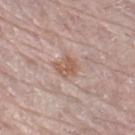<case>
<biopsy_status>not biopsied; imaged during a skin examination</biopsy_status>
<image>
  <source>total-body photography crop</source>
  <field_of_view_mm>15</field_of_view_mm>
</image>
<patient>
  <sex>female</sex>
  <age_approx>65</age_approx>
</patient>
<lesion_size>
  <long_diameter_mm_approx>3.0</long_diameter_mm_approx>
</lesion_size>
<site>left leg</site>
<lighting>white-light</lighting>
</case>